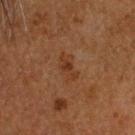Q: Is there a histopathology result?
A: total-body-photography surveillance lesion; no biopsy
Q: What is the anatomic site?
A: the head or neck
Q: How was this image acquired?
A: ~15 mm tile from a whole-body skin photo
Q: Patient demographics?
A: male, about 60 years old